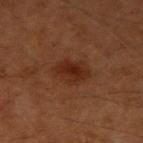notes: catalogued during a skin exam; not biopsied | size: about 5 mm | TBP lesion metrics: an outline eccentricity of about 0.75 (0 = round, 1 = elongated) and a shape-asymmetry score of about 0.2 (0 = symmetric); a nevus-likeness score of about 90/100 | illumination: cross-polarized illumination | image source: ~15 mm tile from a whole-body skin photo | body site: the right upper arm | patient: male, about 60 years old.